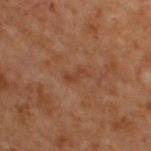{"biopsy_status": "not biopsied; imaged during a skin examination", "patient": {"sex": "male", "age_approx": 70}, "automated_metrics": {"border_irregularity_0_10": 4.0, "color_variation_0_10": 0.0}, "image": {"source": "total-body photography crop", "field_of_view_mm": 15}, "lesion_size": {"long_diameter_mm_approx": 3.0}, "lighting": "cross-polarized", "site": "upper back"}